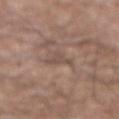Notes:
- acquisition — ~15 mm crop, total-body skin-cancer survey
- location — the abdomen
- illumination — white-light
- lesion diameter — ≈2.5 mm
- subject — male, aged 73 to 77